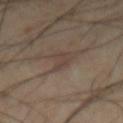<case>
  <biopsy_status>not biopsied; imaged during a skin examination</biopsy_status>
  <image>
    <source>total-body photography crop</source>
    <field_of_view_mm>15</field_of_view_mm>
  </image>
  <automated_metrics>
    <area_mm2_approx>2.0</area_mm2_approx>
    <eccentricity>0.9</eccentricity>
    <shape_asymmetry>0.6</shape_asymmetry>
    <peripheral_color_asymmetry>0.0</peripheral_color_asymmetry>
    <nevus_likeness_0_100>0</nevus_likeness_0_100>
  </automated_metrics>
  <lighting>cross-polarized</lighting>
  <site>mid back</site>
  <patient>
    <sex>male</sex>
    <age_approx>60</age_approx>
  </patient>
  <lesion_size>
    <long_diameter_mm_approx>2.5</long_diameter_mm_approx>
  </lesion_size>
</case>